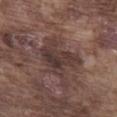Recorded during total-body skin imaging; not selected for excision or biopsy.
Captured under white-light illumination.
A male patient roughly 75 years of age.
A lesion tile, about 15 mm wide, cut from a 3D total-body photograph.
From the abdomen.